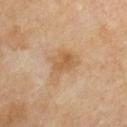This lesion was catalogued during total-body skin photography and was not selected for biopsy.
An algorithmic analysis of the crop reported an outline eccentricity of about 0.7 (0 = round, 1 = elongated). The analysis additionally found a lesion color around L≈56 a*≈18 b*≈36 in CIELAB, about 8 CIELAB-L* units darker than the surrounding skin, and a normalized border contrast of about 6.5.
The lesion's longest dimension is about 4 mm.
The lesion is located on the chest.
Imaged with cross-polarized lighting.
A female patient, about 50 years old.
This image is a 15 mm lesion crop taken from a total-body photograph.